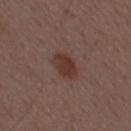Clinical impression:
The lesion was photographed on a routine skin check and not biopsied; there is no pathology result.
Context:
A lesion tile, about 15 mm wide, cut from a 3D total-body photograph. The recorded lesion diameter is about 3.5 mm. From the mid back. A male subject aged approximately 50. Automated image analysis of the tile measured a mean CIELAB color near L≈35 a*≈19 b*≈22, a lesion–skin lightness drop of about 8, and a lesion-to-skin contrast of about 8 (normalized; higher = more distinct). And it measured a border-irregularity index near 2/10.